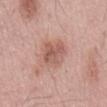Imaged during a routine full-body skin examination; the lesion was not biopsied and no histopathology is available.
About 3.5 mm across.
On the abdomen.
Cropped from a whole-body photographic skin survey; the tile spans about 15 mm.
A male subject, aged approximately 55.
Imaged with white-light lighting.
Automated image analysis of the tile measured a nevus-likeness score of about 10/100 and a lesion-detection confidence of about 100/100.